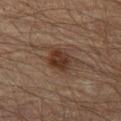biopsy_status: not biopsied; imaged during a skin examination
lighting: cross-polarized
automated_metrics:
  area_mm2_approx: 7.5
  eccentricity: 0.6
  shape_asymmetry: 0.2
  nevus_likeness_0_100: 40
image:
  source: total-body photography crop
  field_of_view_mm: 15
site: leg
lesion_size:
  long_diameter_mm_approx: 3.5
patient:
  sex: male
  age_approx: 60